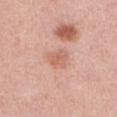notes: no biopsy performed (imaged during a skin exam) | patient: female, roughly 40 years of age | acquisition: ~15 mm tile from a whole-body skin photo | anatomic site: the front of the torso | lesion size: ~2.5 mm (longest diameter) | automated metrics: an area of roughly 5 mm², an eccentricity of roughly 0.6, and two-axis asymmetry of about 0.25; an average lesion color of about L≈63 a*≈24 b*≈29 (CIELAB) and a normalized lesion–skin contrast near 5.5.